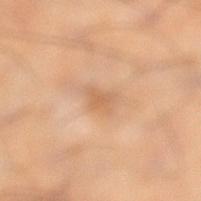Clinical impression: The lesion was tiled from a total-body skin photograph and was not biopsied. Background: This is a cross-polarized tile. Measured at roughly 2.5 mm in maximum diameter. An algorithmic analysis of the crop reported a normalized lesion–skin contrast near 5.5. It also reported an automated nevus-likeness rating near 0 out of 100 and a lesion-detection confidence of about 100/100. On the left lower leg. Cropped from a whole-body photographic skin survey; the tile spans about 15 mm. A male patient aged approximately 40.